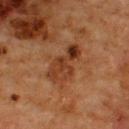Findings:
- workup · imaged on a skin check; not biopsied
- lighting · cross-polarized illumination
- patient · male, aged around 65
- anatomic site · the upper back
- imaging modality · ~15 mm crop, total-body skin-cancer survey